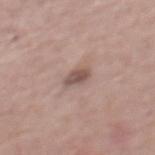Q: Was this lesion biopsied?
A: catalogued during a skin exam; not biopsied
Q: Patient demographics?
A: male, aged approximately 70
Q: What is the imaging modality?
A: 15 mm crop, total-body photography
Q: What is the anatomic site?
A: the mid back